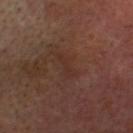biopsy_status: not biopsied; imaged during a skin examination
patient:
  sex: male
  age_approx: 65
site: head or neck
image:
  source: total-body photography crop
  field_of_view_mm: 15
lighting: cross-polarized